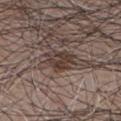The lesion is on the front of the torso.
A male patient about 65 years old.
This is a white-light tile.
Longest diameter approximately 3.5 mm.
Automated image analysis of the tile measured an eccentricity of roughly 0.7 and a shape-asymmetry score of about 0.25 (0 = symmetric). It also reported a lesion color around L≈37 a*≈14 b*≈20 in CIELAB, a lesion–skin lightness drop of about 10, and a normalized lesion–skin contrast near 8.5. And it measured internal color variation of about 4 on a 0–10 scale and a peripheral color-asymmetry measure near 1.5. And it measured a classifier nevus-likeness of about 75/100 and a lesion-detection confidence of about 80/100.
A region of skin cropped from a whole-body photographic capture, roughly 15 mm wide.